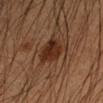From the left forearm.
Measured at roughly 3.5 mm in maximum diameter.
A roughly 15 mm field-of-view crop from a total-body skin photograph.
The patient is a male about 35 years old.